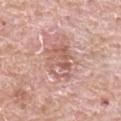The lesion was photographed on a routine skin check and not biopsied; there is no pathology result.
The lesion is located on the back.
A male subject aged 63–67.
About 5.5 mm across.
A lesion tile, about 15 mm wide, cut from a 3D total-body photograph.